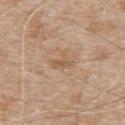Assessment:
Recorded during total-body skin imaging; not selected for excision or biopsy.
Context:
This image is a 15 mm lesion crop taken from a total-body photograph. The lesion is on the upper back. A male subject, roughly 80 years of age.